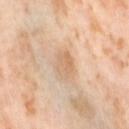Recorded during total-body skin imaging; not selected for excision or biopsy.
Automated image analysis of the tile measured a footprint of about 5.5 mm², an outline eccentricity of about 0.75 (0 = round, 1 = elongated), and two-axis asymmetry of about 0.2. The software also gave a mean CIELAB color near L≈68 a*≈18 b*≈35, a lesion–skin lightness drop of about 8, and a normalized lesion–skin contrast near 5.5. It also reported border irregularity of about 2 on a 0–10 scale, a within-lesion color-variation index near 4.5/10, and a peripheral color-asymmetry measure near 1.5. And it measured a detector confidence of about 100 out of 100 that the crop contains a lesion.
The lesion is on the right thigh.
A female patient in their mid- to late 50s.
A region of skin cropped from a whole-body photographic capture, roughly 15 mm wide.
The tile uses cross-polarized illumination.
Measured at roughly 3.5 mm in maximum diameter.